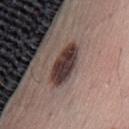• notes — imaged on a skin check; not biopsied
• subject — male, in their mid-40s
• lesion diameter — ≈5.5 mm
• image-analysis metrics — a lesion–skin lightness drop of about 16 and a normalized border contrast of about 13; internal color variation of about 5 on a 0–10 scale and a peripheral color-asymmetry measure near 1.5; an automated nevus-likeness rating near 0 out of 100 and lesion-presence confidence of about 100/100
• body site — the back
• lighting — white-light
• image — ~15 mm crop, total-body skin-cancer survey